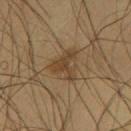Part of a total-body skin-imaging series; this lesion was reviewed on a skin check and was not flagged for biopsy. The lesion's longest dimension is about 3.5 mm. This is a cross-polarized tile. From the leg. The subject is a male aged 63–67. Cropped from a total-body skin-imaging series; the visible field is about 15 mm.